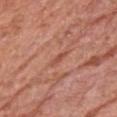Recorded during total-body skin imaging; not selected for excision or biopsy.
On the chest.
The patient is a male roughly 80 years of age.
Automated tile analysis of the lesion measured a lesion area of about 2 mm² and an outline eccentricity of about 0.95 (0 = round, 1 = elongated). The software also gave a border-irregularity rating of about 4/10 and peripheral color asymmetry of about 0. The analysis additionally found an automated nevus-likeness rating near 0 out of 100.
Cropped from a whole-body photographic skin survey; the tile spans about 15 mm.
The tile uses white-light illumination.